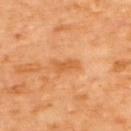follow-up — total-body-photography surveillance lesion; no biopsy
location — the upper back
subject — male, aged approximately 60
image — ~15 mm tile from a whole-body skin photo